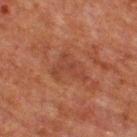biopsy_status: not biopsied; imaged during a skin examination
lesion_size:
  long_diameter_mm_approx: 4.5
image:
  source: total-body photography crop
  field_of_view_mm: 15
automated_metrics:
  border_irregularity_0_10: 6.5
  nevus_likeness_0_100: 0
  lesion_detection_confidence_0_100: 100
lighting: cross-polarized
patient:
  sex: male
  age_approx: 60
site: back